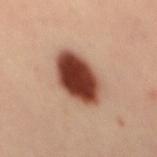biopsy status = no biopsy performed (imaged during a skin exam)
size = ~6.5 mm (longest diameter)
patient = female, roughly 45 years of age
image source = total-body-photography crop, ~15 mm field of view
anatomic site = the mid back